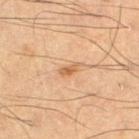biopsy_status: not biopsied; imaged during a skin examination
patient:
  sex: male
  age_approx: 65
lesion_size:
  long_diameter_mm_approx: 2.5
site: left thigh
image:
  source: total-body photography crop
  field_of_view_mm: 15
lighting: cross-polarized